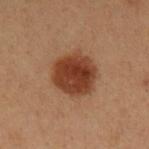Assessment: The lesion was tiled from a total-body skin photograph and was not biopsied. Image and clinical context: Captured under cross-polarized illumination. A 15 mm close-up tile from a total-body photography series done for melanoma screening. Measured at roughly 5 mm in maximum diameter. A male patient aged approximately 55. The lesion is located on the right upper arm.